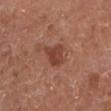Impression: The lesion was photographed on a routine skin check and not biopsied; there is no pathology result. Image and clinical context: Automated image analysis of the tile measured a footprint of about 6 mm², an eccentricity of roughly 0.3, and a shape-asymmetry score of about 0.35 (0 = symmetric). Located on the left lower leg. A male patient roughly 75 years of age. This image is a 15 mm lesion crop taken from a total-body photograph. The lesion's longest dimension is about 3 mm.